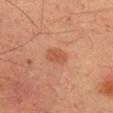  biopsy_status: not biopsied; imaged during a skin examination
  image:
    source: total-body photography crop
    field_of_view_mm: 15
  patient:
    sex: male
    age_approx: 35
  lighting: cross-polarized
  site: right upper arm
  automated_metrics:
    area_mm2_approx: 4.5
    eccentricity: 0.75
    shape_asymmetry: 0.2
    cielab_L: 49
    cielab_a: 25
    cielab_b: 33
    vs_skin_darker_L: 7.0
    vs_skin_contrast_norm: 6.0
    border_irregularity_0_10: 2.0
    color_variation_0_10: 2.0
    peripheral_color_asymmetry: 0.5
  lesion_size:
    long_diameter_mm_approx: 3.0